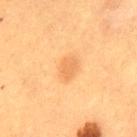Clinical summary:
A 15 mm crop from a total-body photograph taken for skin-cancer surveillance. The lesion is located on the back. Imaged with cross-polarized lighting. A male subject, aged around 50.